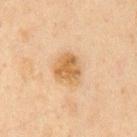Located on the front of the torso. A roughly 15 mm field-of-view crop from a total-body skin photograph. A male subject, roughly 60 years of age. Imaged with cross-polarized lighting. The recorded lesion diameter is about 4 mm.